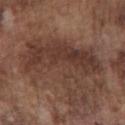Impression: The lesion was tiled from a total-body skin photograph and was not biopsied. Image and clinical context: The lesion-visualizer software estimated a mean CIELAB color near L≈37 a*≈18 b*≈24 and about 8 CIELAB-L* units darker than the surrounding skin. It also reported a classifier nevus-likeness of about 5/100 and lesion-presence confidence of about 55/100. Approximately 9.5 mm at its widest. On the chest. The patient is a male aged 73 to 77. Imaged with white-light lighting. A close-up tile cropped from a whole-body skin photograph, about 15 mm across.